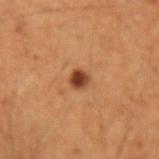  biopsy_status: not biopsied; imaged during a skin examination
  lighting: cross-polarized
  automated_metrics:
    cielab_L: 41
    cielab_a: 24
    cielab_b: 34
    vs_skin_contrast_norm: 11.0
  patient:
    sex: male
    age_approx: 50
  lesion_size:
    long_diameter_mm_approx: 2.0
  site: left forearm
  image:
    source: total-body photography crop
    field_of_view_mm: 15